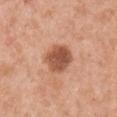Context:
A male subject, aged around 55. On the right upper arm. A 15 mm close-up tile from a total-body photography series done for melanoma screening. Captured under white-light illumination. Automated tile analysis of the lesion measured an average lesion color of about L≈53 a*≈25 b*≈32 (CIELAB), a lesion–skin lightness drop of about 14, and a lesion-to-skin contrast of about 9.5 (normalized; higher = more distinct).